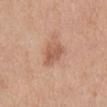Findings:
* follow-up — catalogued during a skin exam; not biopsied
* subject — female, about 40 years old
* diameter — about 3.5 mm
* image-analysis metrics — a border-irregularity index near 3/10, a color-variation rating of about 2.5/10, and radial color variation of about 1; a detector confidence of about 100 out of 100 that the crop contains a lesion
* anatomic site — the abdomen
* imaging modality — total-body-photography crop, ~15 mm field of view